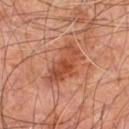Q: Is there a histopathology result?
A: catalogued during a skin exam; not biopsied
Q: What are the patient's age and sex?
A: male, aged approximately 60
Q: Illumination type?
A: cross-polarized
Q: What is the imaging modality?
A: ~15 mm tile from a whole-body skin photo
Q: What is the lesion's diameter?
A: about 6 mm
Q: Where on the body is the lesion?
A: the right leg
Q: Automated lesion metrics?
A: a footprint of about 13 mm²; a border-irregularity rating of about 5/10, internal color variation of about 5 on a 0–10 scale, and peripheral color asymmetry of about 2